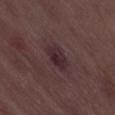<case>
  <patient>
    <sex>male</sex>
    <age_approx>50</age_approx>
  </patient>
  <lighting>white-light</lighting>
  <automated_metrics>
    <border_irregularity_0_10>2.0</border_irregularity_0_10>
    <color_variation_0_10>3.5</color_variation_0_10>
    <peripheral_color_asymmetry>1.0</peripheral_color_asymmetry>
    <nevus_likeness_0_100>5</nevus_likeness_0_100>
    <lesion_detection_confidence_0_100>100</lesion_detection_confidence_0_100>
  </automated_metrics>
  <image>
    <source>total-body photography crop</source>
    <field_of_view_mm>15</field_of_view_mm>
  </image>
  <lesion_size>
    <long_diameter_mm_approx>3.5</long_diameter_mm_approx>
  </lesion_size>
</case>